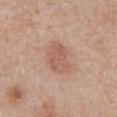The subject is a male approximately 70 years of age.
A close-up tile cropped from a whole-body skin photograph, about 15 mm across.
The recorded lesion diameter is about 4.5 mm.
Located on the abdomen.